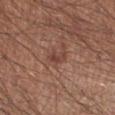The lesion was tiled from a total-body skin photograph and was not biopsied.
The patient is a male approximately 45 years of age.
Captured under white-light illumination.
About 2.5 mm across.
The lesion-visualizer software estimated about 8 CIELAB-L* units darker than the surrounding skin and a lesion-to-skin contrast of about 6.5 (normalized; higher = more distinct). It also reported an automated nevus-likeness rating near 5 out of 100.
Cropped from a whole-body photographic skin survey; the tile spans about 15 mm.
On the right lower leg.